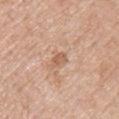Part of a total-body skin-imaging series; this lesion was reviewed on a skin check and was not flagged for biopsy. On the upper back. A male patient, about 70 years old. This image is a 15 mm lesion crop taken from a total-body photograph.